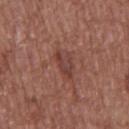Assessment:
No biopsy was performed on this lesion — it was imaged during a full skin examination and was not determined to be concerning.
Acquisition and patient details:
The lesion is located on the chest. Captured under white-light illumination. This image is a 15 mm lesion crop taken from a total-body photograph. A male subject aged 73–77.